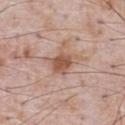Q: Was a biopsy performed?
A: total-body-photography surveillance lesion; no biopsy
Q: What is the imaging modality?
A: 15 mm crop, total-body photography
Q: Automated lesion metrics?
A: border irregularity of about 3.5 on a 0–10 scale and a within-lesion color-variation index near 4/10; an automated nevus-likeness rating near 70 out of 100 and lesion-presence confidence of about 100/100
Q: Lesion size?
A: ≈4 mm
Q: Patient demographics?
A: male, aged around 70
Q: Where on the body is the lesion?
A: the chest